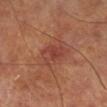Q: Was this lesion biopsied?
A: total-body-photography surveillance lesion; no biopsy
Q: How was this image acquired?
A: total-body-photography crop, ~15 mm field of view
Q: How large is the lesion?
A: about 3.5 mm
Q: How was the tile lit?
A: cross-polarized illumination
Q: What are the patient's age and sex?
A: male, approximately 70 years of age
Q: Where on the body is the lesion?
A: the right lower leg